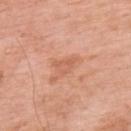Captured during whole-body skin photography for melanoma surveillance; the lesion was not biopsied.
The lesion-visualizer software estimated a lesion area of about 3.5 mm² and a shape eccentricity near 0.65. The software also gave a border-irregularity rating of about 4.5/10, a color-variation rating of about 2/10, and a peripheral color-asymmetry measure near 0.5.
This is a white-light tile.
The lesion is located on the upper back.
A 15 mm close-up extracted from a 3D total-body photography capture.
About 2.5 mm across.
The subject is a male roughly 60 years of age.